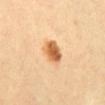No biopsy was performed on this lesion — it was imaged during a full skin examination and was not determined to be concerning. The patient is aged approximately 60. A 15 mm close-up extracted from a 3D total-body photography capture. Longest diameter approximately 3 mm. The lesion is on the abdomen. The tile uses cross-polarized illumination.